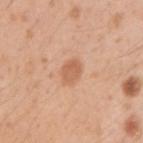The lesion was tiled from a total-body skin photograph and was not biopsied. The subject is a male aged 28–32. On the right upper arm. A 15 mm crop from a total-body photograph taken for skin-cancer surveillance. The tile uses white-light illumination. An algorithmic analysis of the crop reported a footprint of about 5 mm², a shape eccentricity near 0.5, and a shape-asymmetry score of about 0.15 (0 = symmetric). It also reported an average lesion color of about L≈61 a*≈23 b*≈34 (CIELAB). And it measured a nevus-likeness score of about 60/100 and a lesion-detection confidence of about 100/100.